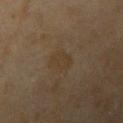biopsy status = catalogued during a skin exam; not biopsied
acquisition = 15 mm crop, total-body photography
patient = female, aged around 60
diameter = ~3 mm (longest diameter)
illumination = cross-polarized illumination
body site = the left upper arm